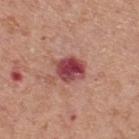Q: Is there a histopathology result?
A: imaged on a skin check; not biopsied
Q: Where on the body is the lesion?
A: the back
Q: Lesion size?
A: about 3.5 mm
Q: What did automated image analysis measure?
A: a lesion–skin lightness drop of about 16 and a lesion-to-skin contrast of about 11.5 (normalized; higher = more distinct)
Q: Illumination type?
A: white-light
Q: What is the imaging modality?
A: total-body-photography crop, ~15 mm field of view
Q: Who is the patient?
A: male, aged around 70